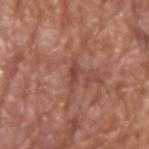Impression: This lesion was catalogued during total-body skin photography and was not selected for biopsy. Clinical summary: A male patient aged around 75. The recorded lesion diameter is about 3 mm. Located on the left upper arm. A 15 mm close-up extracted from a 3D total-body photography capture. Automated image analysis of the tile measured border irregularity of about 4.5 on a 0–10 scale and a color-variation rating of about 0/10. It also reported an automated nevus-likeness rating near 0 out of 100 and a lesion-detection confidence of about 90/100.